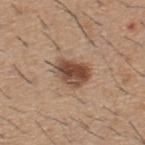follow-up — no biopsy performed (imaged during a skin exam) | imaging modality — ~15 mm crop, total-body skin-cancer survey | diameter — ~4 mm (longest diameter) | patient — male, approximately 60 years of age | tile lighting — white-light | body site — the upper back.